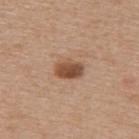Notes:
- biopsy status · catalogued during a skin exam; not biopsied
- lesion diameter · ≈3 mm
- image source · total-body-photography crop, ~15 mm field of view
- illumination · white-light illumination
- automated metrics · an area of roughly 6 mm² and a shape-asymmetry score of about 0.15 (0 = symmetric); a lesion color around L≈49 a*≈21 b*≈31 in CIELAB, about 14 CIELAB-L* units darker than the surrounding skin, and a normalized border contrast of about 10; a nevus-likeness score of about 90/100 and a lesion-detection confidence of about 100/100
- patient · female, in their mid-60s
- body site · the upper back